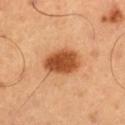<lesion>
<biopsy_status>not biopsied; imaged during a skin examination</biopsy_status>
<image>
  <source>total-body photography crop</source>
  <field_of_view_mm>15</field_of_view_mm>
</image>
<lighting>cross-polarized</lighting>
<site>left thigh</site>
<automated_metrics>
  <nevus_likeness_0_100>100</nevus_likeness_0_100>
</automated_metrics>
<patient>
  <sex>male</sex>
  <age_approx>50</age_approx>
</patient>
<lesion_size>
  <long_diameter_mm_approx>5.0</long_diameter_mm_approx>
</lesion_size>
</lesion>